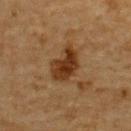Q: Is there a histopathology result?
A: catalogued during a skin exam; not biopsied